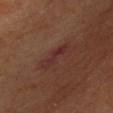Notes:
• notes · catalogued during a skin exam; not biopsied
• site · the chest
• diameter · ≈4 mm
• illumination · cross-polarized illumination
• image source · total-body-photography crop, ~15 mm field of view
• subject · male, aged 58–62